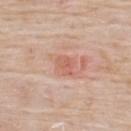Clinical impression:
No biopsy was performed on this lesion — it was imaged during a full skin examination and was not determined to be concerning.
Image and clinical context:
A male patient roughly 75 years of age. The recorded lesion diameter is about 2.5 mm. Automated tile analysis of the lesion measured an area of roughly 4 mm² and a symmetry-axis asymmetry near 0.25. And it measured an average lesion color of about L≈61 a*≈25 b*≈30 (CIELAB), about 8 CIELAB-L* units darker than the surrounding skin, and a normalized lesion–skin contrast near 5.5. And it measured a border-irregularity index near 2.5/10, a within-lesion color-variation index near 3/10, and a peripheral color-asymmetry measure near 1. The lesion is located on the upper back. This image is a 15 mm lesion crop taken from a total-body photograph.